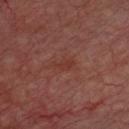acquisition: 15 mm crop, total-body photography; site: the chest; patient: male, aged around 65.